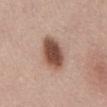biopsy status=catalogued during a skin exam; not biopsied
anatomic site=the abdomen
subject=male, approximately 50 years of age
diameter=about 5.5 mm
automated metrics=an area of roughly 13 mm² and two-axis asymmetry of about 0.15; a lesion color around L≈50 a*≈20 b*≈26 in CIELAB, a lesion–skin lightness drop of about 17, and a normalized lesion–skin contrast near 11.5; a color-variation rating of about 5/10 and radial color variation of about 1.5; an automated nevus-likeness rating near 100 out of 100 and a detector confidence of about 100 out of 100 that the crop contains a lesion
illumination=white-light illumination
image source=~15 mm crop, total-body skin-cancer survey